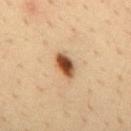Impression: This lesion was catalogued during total-body skin photography and was not selected for biopsy. Acquisition and patient details: The recorded lesion diameter is about 3.5 mm. A 15 mm close-up tile from a total-body photography series done for melanoma screening. The total-body-photography lesion software estimated an average lesion color of about L≈48 a*≈20 b*≈33 (CIELAB), about 17 CIELAB-L* units darker than the surrounding skin, and a normalized border contrast of about 12. The analysis additionally found a border-irregularity index near 2/10, a within-lesion color-variation index near 7/10, and a peripheral color-asymmetry measure near 2. A male subject, in their mid-30s. From the mid back.